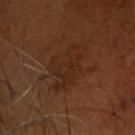Clinical impression:
The lesion was photographed on a routine skin check and not biopsied; there is no pathology result.
Clinical summary:
Measured at roughly 5.5 mm in maximum diameter. A 15 mm close-up tile from a total-body photography series done for melanoma screening. This is a cross-polarized tile. Located on the head or neck. A male subject, roughly 65 years of age.